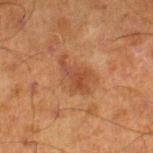Clinical impression:
No biopsy was performed on this lesion — it was imaged during a full skin examination and was not determined to be concerning.
Image and clinical context:
The lesion is located on the right lower leg. This is a cross-polarized tile. The lesion-visualizer software estimated an area of roughly 10 mm². The software also gave a normalized lesion–skin contrast near 6. It also reported border irregularity of about 4.5 on a 0–10 scale and a within-lesion color-variation index near 4.5/10. The patient is a male approximately 70 years of age. A lesion tile, about 15 mm wide, cut from a 3D total-body photograph.